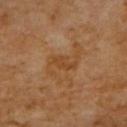Imaged during a routine full-body skin examination; the lesion was not biopsied and no histopathology is available.
Cropped from a whole-body photographic skin survey; the tile spans about 15 mm.
A female patient, aged approximately 65.
Located on the upper back.
The tile uses cross-polarized illumination.
Approximately 3 mm at its widest.